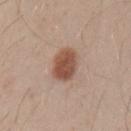workup: no biopsy performed (imaged during a skin exam); patient: male, roughly 30 years of age; imaging modality: total-body-photography crop, ~15 mm field of view; tile lighting: white-light; lesion size: about 4 mm; location: the left upper arm.